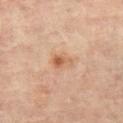No biopsy was performed on this lesion — it was imaged during a full skin examination and was not determined to be concerning.
On the right leg.
A female patient, aged 63 to 67.
Measured at roughly 3 mm in maximum diameter.
This is a cross-polarized tile.
A close-up tile cropped from a whole-body skin photograph, about 15 mm across.